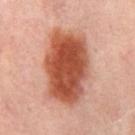  biopsy_status: not biopsied; imaged during a skin examination
  image:
    source: total-body photography crop
    field_of_view_mm: 15
  automated_metrics:
    cielab_L: 50
    cielab_a: 28
    cielab_b: 32
    vs_skin_darker_L: 17.0
  patient:
    sex: female
    age_approx: 50
  site: left thigh
  lesion_size:
    long_diameter_mm_approx: 9.0